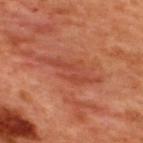biopsy status: catalogued during a skin exam; not biopsied | body site: the upper back | lesion size: about 7 mm | subject: male, roughly 50 years of age | acquisition: ~15 mm tile from a whole-body skin photo.